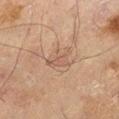Captured under cross-polarized illumination. An algorithmic analysis of the crop reported a mean CIELAB color near L≈46 a*≈16 b*≈24, about 6 CIELAB-L* units darker than the surrounding skin, and a normalized border contrast of about 4.5. The recorded lesion diameter is about 3.5 mm. A male subject roughly 70 years of age. From the left thigh. A roughly 15 mm field-of-view crop from a total-body skin photograph.